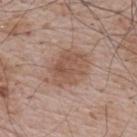Impression:
Part of a total-body skin-imaging series; this lesion was reviewed on a skin check and was not flagged for biopsy.
Context:
The lesion is located on the upper back. A male patient roughly 65 years of age. The lesion's longest dimension is about 5.5 mm. A close-up tile cropped from a whole-body skin photograph, about 15 mm across. Captured under white-light illumination.